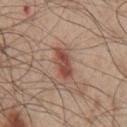Captured during whole-body skin photography for melanoma surveillance; the lesion was not biopsied. On the chest. A male subject aged around 55. A 15 mm close-up extracted from a 3D total-body photography capture. An algorithmic analysis of the crop reported a lesion area of about 7 mm², a shape eccentricity near 0.85, and a shape-asymmetry score of about 0.25 (0 = symmetric). The analysis additionally found border irregularity of about 3 on a 0–10 scale, a within-lesion color-variation index near 3.5/10, and a peripheral color-asymmetry measure near 1. It also reported an automated nevus-likeness rating near 35 out of 100. Captured under white-light illumination. The recorded lesion diameter is about 4 mm.